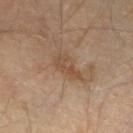Findings:
– follow-up: catalogued during a skin exam; not biopsied
– lighting: cross-polarized
– site: the left lower leg
– automated metrics: two-axis asymmetry of about 0.3; a nevus-likeness score of about 0/100
– image: ~15 mm crop, total-body skin-cancer survey
– diameter: ~4 mm (longest diameter)
– patient: male, approximately 45 years of age